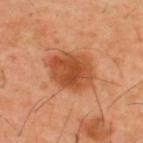The lesion was tiled from a total-body skin photograph and was not biopsied. A roughly 15 mm field-of-view crop from a total-body skin photograph. On the back. Captured under cross-polarized illumination. The lesion's longest dimension is about 5.5 mm. A male patient, roughly 40 years of age.